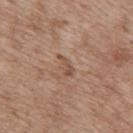biopsy status: total-body-photography surveillance lesion; no biopsy | lesion size: about 3 mm | patient: male, aged 68 to 72 | lighting: white-light illumination | image-analysis metrics: a lesion area of about 2.5 mm², an eccentricity of roughly 0.95, and two-axis asymmetry of about 0.5 | acquisition: total-body-photography crop, ~15 mm field of view | anatomic site: the back.